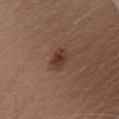This lesion was catalogued during total-body skin photography and was not selected for biopsy. Captured under white-light illumination. A male patient in their mid- to late 50s. On the front of the torso. Cropped from a whole-body photographic skin survey; the tile spans about 15 mm. An algorithmic analysis of the crop reported a shape eccentricity near 0.75. And it measured a border-irregularity rating of about 2/10, internal color variation of about 3.5 on a 0–10 scale, and radial color variation of about 1. The analysis additionally found a classifier nevus-likeness of about 85/100.